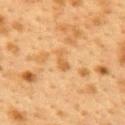Impression: Captured during whole-body skin photography for melanoma surveillance; the lesion was not biopsied. Acquisition and patient details: A lesion tile, about 15 mm wide, cut from a 3D total-body photograph. A female patient, aged 38 to 42. On the upper back. About 2.5 mm across. Automated image analysis of the tile measured a lesion color around L≈52 a*≈19 b*≈39 in CIELAB, a lesion–skin lightness drop of about 7, and a lesion-to-skin contrast of about 6 (normalized; higher = more distinct). It also reported a border-irregularity index near 3.5/10 and a within-lesion color-variation index near 0.5/10. Imaged with cross-polarized lighting.